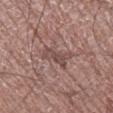Clinical summary: The lesion is located on the right lower leg. A male patient, in their mid- to late 70s. A lesion tile, about 15 mm wide, cut from a 3D total-body photograph.